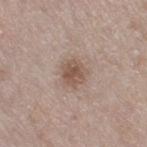| field | value |
|---|---|
| follow-up | catalogued during a skin exam; not biopsied |
| body site | the right thigh |
| patient | female, about 60 years old |
| image source | ~15 mm tile from a whole-body skin photo |